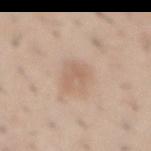Captured during whole-body skin photography for melanoma surveillance; the lesion was not biopsied.
The subject is a male approximately 30 years of age.
From the abdomen.
The tile uses white-light illumination.
The recorded lesion diameter is about 3 mm.
Automated tile analysis of the lesion measured a classifier nevus-likeness of about 0/100 and a lesion-detection confidence of about 100/100.
A 15 mm close-up extracted from a 3D total-body photography capture.